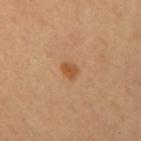Part of a total-body skin-imaging series; this lesion was reviewed on a skin check and was not flagged for biopsy.
A female subject.
The lesion is located on the mid back.
Imaged with cross-polarized lighting.
Cropped from a total-body skin-imaging series; the visible field is about 15 mm.